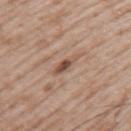Q: Was this lesion biopsied?
A: imaged on a skin check; not biopsied
Q: Who is the patient?
A: male, aged approximately 50
Q: What is the anatomic site?
A: the upper back
Q: What kind of image is this?
A: ~15 mm tile from a whole-body skin photo